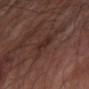biopsy_status: not biopsied; imaged during a skin examination
lighting: cross-polarized
patient:
  sex: male
  age_approx: 65
site: left forearm
image:
  source: total-body photography crop
  field_of_view_mm: 15
lesion_size:
  long_diameter_mm_approx: 3.0